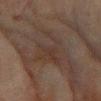Case summary:
* follow-up — catalogued during a skin exam; not biopsied
* lighting — cross-polarized illumination
* imaging modality — total-body-photography crop, ~15 mm field of view
* location — the right forearm
* patient — female, aged approximately 80